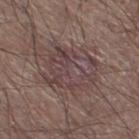Case summary:
* biopsy status — catalogued during a skin exam; not biopsied
* size — ≈6.5 mm
* automated lesion analysis — a mean CIELAB color near L≈42 a*≈16 b*≈16, roughly 6 lightness units darker than nearby skin, and a normalized lesion–skin contrast near 6; a border-irregularity index near 4.5/10, a color-variation rating of about 6/10, and radial color variation of about 2; an automated nevus-likeness rating near 0 out of 100 and a detector confidence of about 90 out of 100 that the crop contains a lesion
* imaging modality — ~15 mm crop, total-body skin-cancer survey
* location — the left lower leg
* subject — male, aged 48 to 52
* tile lighting — white-light